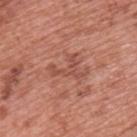| field | value |
|---|---|
| workup | no biopsy performed (imaged during a skin exam) |
| location | the upper back |
| patient | male, aged approximately 70 |
| lesion size | about 4.5 mm |
| acquisition | 15 mm crop, total-body photography |